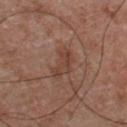An algorithmic analysis of the crop reported a mean CIELAB color near L≈42 a*≈20 b*≈27 and a normalized lesion–skin contrast near 6. The analysis additionally found a classifier nevus-likeness of about 5/100 and a detector confidence of about 100 out of 100 that the crop contains a lesion. This image is a 15 mm lesion crop taken from a total-body photograph. Captured under white-light illumination. A male patient, aged 53 to 57. From the front of the torso. Measured at roughly 4 mm in maximum diameter.